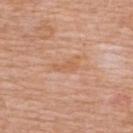Findings:
– notes: catalogued during a skin exam; not biopsied